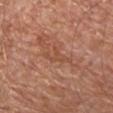Clinical impression: Part of a total-body skin-imaging series; this lesion was reviewed on a skin check and was not flagged for biopsy. Background: The patient is a male aged 63 to 67. This is a white-light tile. An algorithmic analysis of the crop reported a footprint of about 4 mm², a shape eccentricity near 0.85, and a symmetry-axis asymmetry near 0.6. And it measured a lesion color around L≈50 a*≈24 b*≈32 in CIELAB and a lesion-to-skin contrast of about 5 (normalized; higher = more distinct). And it measured a nevus-likeness score of about 0/100 and a lesion-detection confidence of about 60/100. The recorded lesion diameter is about 3.5 mm. A region of skin cropped from a whole-body photographic capture, roughly 15 mm wide. On the chest.